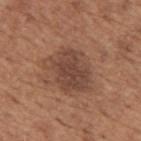Case summary:
* follow-up: imaged on a skin check; not biopsied
* image: ~15 mm crop, total-body skin-cancer survey
* lesion size: ≈5.5 mm
* site: the upper back
* patient: male, roughly 65 years of age
* illumination: white-light